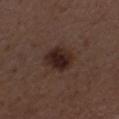The lesion was photographed on a routine skin check and not biopsied; there is no pathology result.
The patient is a female about 50 years old.
The tile uses white-light illumination.
Located on the right forearm.
A region of skin cropped from a whole-body photographic capture, roughly 15 mm wide.
The total-body-photography lesion software estimated an eccentricity of roughly 0.65 and two-axis asymmetry of about 0.15. It also reported a mean CIELAB color near L≈23 a*≈16 b*≈19 and a lesion-to-skin contrast of about 11.5 (normalized; higher = more distinct). And it measured a classifier nevus-likeness of about 85/100 and a detector confidence of about 100 out of 100 that the crop contains a lesion.
The recorded lesion diameter is about 4 mm.